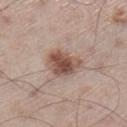Context: The lesion is on the left lower leg. An algorithmic analysis of the crop reported a footprint of about 10 mm², an outline eccentricity of about 0.6 (0 = round, 1 = elongated), and a symmetry-axis asymmetry near 0.3. The analysis additionally found a lesion color around L≈51 a*≈19 b*≈25 in CIELAB, roughly 13 lightness units darker than nearby skin, and a normalized border contrast of about 9.5. And it measured border irregularity of about 2.5 on a 0–10 scale, a color-variation rating of about 5/10, and a peripheral color-asymmetry measure near 1.5. The software also gave a lesion-detection confidence of about 100/100. Captured under white-light illumination. The patient is a male roughly 60 years of age. Measured at roughly 4 mm in maximum diameter. A lesion tile, about 15 mm wide, cut from a 3D total-body photograph.